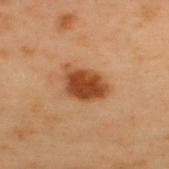Part of a total-body skin-imaging series; this lesion was reviewed on a skin check and was not flagged for biopsy. A lesion tile, about 15 mm wide, cut from a 3D total-body photograph. The patient is a male aged around 55. Approximately 4.5 mm at its widest. On the upper back. Captured under cross-polarized illumination.